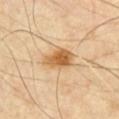Impression:
Part of a total-body skin-imaging series; this lesion was reviewed on a skin check and was not flagged for biopsy.
Clinical summary:
Measured at roughly 4 mm in maximum diameter. A lesion tile, about 15 mm wide, cut from a 3D total-body photograph. This is a cross-polarized tile. A male patient in their mid- to late 60s. The lesion is on the chest. Automated image analysis of the tile measured a mean CIELAB color near L≈61 a*≈19 b*≈41, a lesion–skin lightness drop of about 12, and a normalized border contrast of about 9. It also reported a border-irregularity rating of about 2/10 and radial color variation of about 1.5. The software also gave a detector confidence of about 100 out of 100 that the crop contains a lesion.